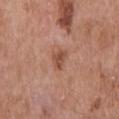| feature | finding |
|---|---|
| notes | imaged on a skin check; not biopsied |
| acquisition | total-body-photography crop, ~15 mm field of view |
| location | the upper back |
| patient | male, roughly 65 years of age |